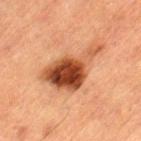Captured under cross-polarized illumination. From the left thigh. The patient is a male about 85 years old or older. Cropped from a total-body skin-imaging series; the visible field is about 15 mm. Longest diameter approximately 7 mm.